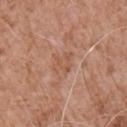Impression:
No biopsy was performed on this lesion — it was imaged during a full skin examination and was not determined to be concerning.
Clinical summary:
Measured at roughly 3 mm in maximum diameter. A male subject, in their 60s. Automated image analysis of the tile measured two-axis asymmetry of about 0.4. The analysis additionally found an average lesion color of about L≈55 a*≈22 b*≈31 (CIELAB), a lesion–skin lightness drop of about 6, and a normalized lesion–skin contrast near 5. The software also gave a border-irregularity index near 4/10, a color-variation rating of about 2/10, and radial color variation of about 0.5. Captured under white-light illumination. On the arm. A region of skin cropped from a whole-body photographic capture, roughly 15 mm wide.